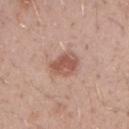Q: Was this lesion biopsied?
A: no biopsy performed (imaged during a skin exam)
Q: What did automated image analysis measure?
A: a lesion color around L≈55 a*≈22 b*≈27 in CIELAB, about 11 CIELAB-L* units darker than the surrounding skin, and a normalized lesion–skin contrast near 7.5; border irregularity of about 2 on a 0–10 scale and a peripheral color-asymmetry measure near 1.5; a nevus-likeness score of about 80/100 and a detector confidence of about 100 out of 100 that the crop contains a lesion
Q: Who is the patient?
A: male, aged around 30
Q: Lesion size?
A: ≈3 mm
Q: Where on the body is the lesion?
A: the right forearm
Q: How was this image acquired?
A: 15 mm crop, total-body photography
Q: What lighting was used for the tile?
A: white-light